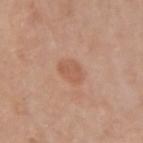Q: Is there a histopathology result?
A: imaged on a skin check; not biopsied
Q: What are the patient's age and sex?
A: female, aged 33 to 37
Q: Lesion location?
A: the left forearm
Q: Illumination type?
A: white-light
Q: How was this image acquired?
A: 15 mm crop, total-body photography
Q: Lesion size?
A: ~3 mm (longest diameter)